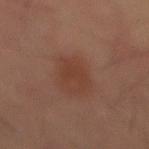Clinical impression: This lesion was catalogued during total-body skin photography and was not selected for biopsy. Clinical summary: An algorithmic analysis of the crop reported a footprint of about 5 mm², an eccentricity of roughly 0.65, and a symmetry-axis asymmetry near 0.4. The software also gave an average lesion color of about L≈32 a*≈19 b*≈24 (CIELAB), a lesion–skin lightness drop of about 5, and a normalized lesion–skin contrast near 5.5. It also reported a classifier nevus-likeness of about 50/100 and a detector confidence of about 100 out of 100 that the crop contains a lesion. A male patient in their mid-50s. A region of skin cropped from a whole-body photographic capture, roughly 15 mm wide. The tile uses cross-polarized illumination. Located on the abdomen. Measured at roughly 3 mm in maximum diameter.